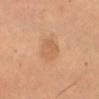Q: Was a biopsy performed?
A: catalogued during a skin exam; not biopsied
Q: Who is the patient?
A: female, aged around 65
Q: Lesion location?
A: the abdomen
Q: What kind of image is this?
A: total-body-photography crop, ~15 mm field of view